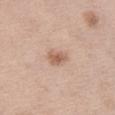Q: Was this lesion biopsied?
A: total-body-photography surveillance lesion; no biopsy
Q: Where on the body is the lesion?
A: the left thigh
Q: What kind of image is this?
A: 15 mm crop, total-body photography
Q: Illumination type?
A: white-light
Q: What are the patient's age and sex?
A: female, aged 38 to 42